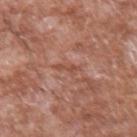Impression:
Recorded during total-body skin imaging; not selected for excision or biopsy.
Background:
About 2.5 mm across. The tile uses white-light illumination. A male patient in their 60s. A 15 mm close-up tile from a total-body photography series done for melanoma screening. The lesion is located on the left forearm.